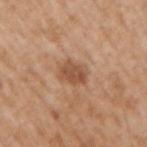Assessment:
Captured during whole-body skin photography for melanoma surveillance; the lesion was not biopsied.
Background:
This is a white-light tile. This image is a 15 mm lesion crop taken from a total-body photograph. Automated tile analysis of the lesion measured an area of roughly 6 mm², an eccentricity of roughly 0.65, and a symmetry-axis asymmetry near 0.2. It also reported a lesion color around L≈51 a*≈21 b*≈33 in CIELAB, about 11 CIELAB-L* units darker than the surrounding skin, and a normalized border contrast of about 7.5. And it measured a border-irregularity rating of about 2/10 and a peripheral color-asymmetry measure near 1. The analysis additionally found an automated nevus-likeness rating near 25 out of 100. The lesion is located on the right upper arm. The patient is a male aged around 65.